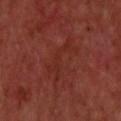Clinical impression:
Imaged during a routine full-body skin examination; the lesion was not biopsied and no histopathology is available.
Image and clinical context:
On the upper back. A male subject aged around 65. A lesion tile, about 15 mm wide, cut from a 3D total-body photograph.